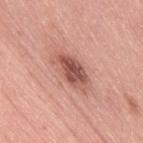{"biopsy_status": "not biopsied; imaged during a skin examination", "lighting": "white-light", "image": {"source": "total-body photography crop", "field_of_view_mm": 15}, "site": "right thigh", "automated_metrics": {"area_mm2_approx": 10.0, "cielab_L": 54, "cielab_a": 25, "cielab_b": 27, "vs_skin_contrast_norm": 9.0, "border_irregularity_0_10": 2.5, "color_variation_0_10": 6.0, "peripheral_color_asymmetry": 2.0, "nevus_likeness_0_100": 10, "lesion_detection_confidence_0_100": 100}, "patient": {"sex": "female", "age_approx": 40}}